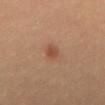{
  "biopsy_status": "not biopsied; imaged during a skin examination",
  "lesion_size": {
    "long_diameter_mm_approx": 2.0
  },
  "patient": {
    "sex": "female",
    "age_approx": 30
  },
  "image": {
    "source": "total-body photography crop",
    "field_of_view_mm": 15
  },
  "site": "mid back"
}